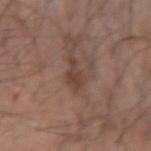biopsy status = no biopsy performed (imaged during a skin exam) | lesion size = ~3.5 mm (longest diameter) | subject = male, approximately 70 years of age | body site = the arm | illumination = white-light | acquisition = ~15 mm crop, total-body skin-cancer survey.